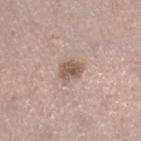Recorded during total-body skin imaging; not selected for excision or biopsy.
Located on the right lower leg.
Automated image analysis of the tile measured a lesion area of about 5.5 mm², an eccentricity of roughly 0.65, and two-axis asymmetry of about 0.2. And it measured a color-variation rating of about 3.5/10 and a peripheral color-asymmetry measure near 1. The software also gave a nevus-likeness score of about 50/100 and a lesion-detection confidence of about 100/100.
About 3 mm across.
A roughly 15 mm field-of-view crop from a total-body skin photograph.
This is a white-light tile.
A female subject, in their mid- to late 20s.